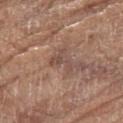Recorded during total-body skin imaging; not selected for excision or biopsy. From the right thigh. The tile uses white-light illumination. An algorithmic analysis of the crop reported a lesion color around L≈48 a*≈18 b*≈24 in CIELAB, about 7 CIELAB-L* units darker than the surrounding skin, and a lesion-to-skin contrast of about 6 (normalized; higher = more distinct). It also reported a border-irregularity rating of about 7/10, a within-lesion color-variation index near 1/10, and peripheral color asymmetry of about 0. It also reported lesion-presence confidence of about 90/100. A close-up tile cropped from a whole-body skin photograph, about 15 mm across. The lesion's longest dimension is about 3 mm. A female subject, about 75 years old.